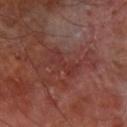Clinical impression: The lesion was photographed on a routine skin check and not biopsied; there is no pathology result. Context: Captured under cross-polarized illumination. The lesion's longest dimension is about 3.5 mm. A male subject about 65 years old. This image is a 15 mm lesion crop taken from a total-body photograph. On the right forearm. The lesion-visualizer software estimated a mean CIELAB color near L≈32 a*≈25 b*≈23, a lesion–skin lightness drop of about 5, and a normalized border contrast of about 5. And it measured border irregularity of about 5 on a 0–10 scale.